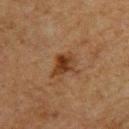{
  "biopsy_status": "not biopsied; imaged during a skin examination"
}